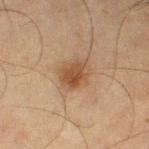This lesion was catalogued during total-body skin photography and was not selected for biopsy. On the left thigh. A lesion tile, about 15 mm wide, cut from a 3D total-body photograph. Measured at roughly 3.5 mm in maximum diameter. The lesion-visualizer software estimated an average lesion color of about L≈41 a*≈16 b*≈29 (CIELAB), roughly 9 lightness units darker than nearby skin, and a normalized border contrast of about 8. The software also gave a border-irregularity index near 2/10, a color-variation rating of about 3/10, and a peripheral color-asymmetry measure near 1. The patient is a male in their mid-60s. The tile uses cross-polarized illumination.